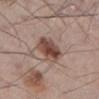Assessment:
Recorded during total-body skin imaging; not selected for excision or biopsy.
Background:
Cropped from a whole-body photographic skin survey; the tile spans about 15 mm. The subject is a male aged 58–62. From the left lower leg.